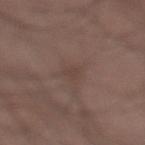workup = catalogued during a skin exam; not biopsied
imaging modality = ~15 mm crop, total-body skin-cancer survey
tile lighting = white-light illumination
subject = male, in their mid- to late 30s
lesion diameter = ~2.5 mm (longest diameter)
body site = the left lower leg